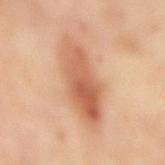This lesion was catalogued during total-body skin photography and was not selected for biopsy.
The lesion's longest dimension is about 7.5 mm.
The lesion is located on the back.
An algorithmic analysis of the crop reported an eccentricity of roughly 0.9 and a shape-asymmetry score of about 0.15 (0 = symmetric). It also reported a detector confidence of about 100 out of 100 that the crop contains a lesion.
Imaged with cross-polarized lighting.
Cropped from a whole-body photographic skin survey; the tile spans about 15 mm.
A female patient, aged around 55.